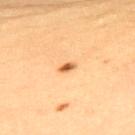Impression:
Captured during whole-body skin photography for melanoma surveillance; the lesion was not biopsied.
Background:
The subject is a female roughly 35 years of age. From the upper back. Approximately 2 mm at its widest. Automated tile analysis of the lesion measured an area of roughly 2 mm² and an outline eccentricity of about 0.85 (0 = round, 1 = elongated). It also reported an average lesion color of about L≈63 a*≈25 b*≈45 (CIELAB), roughly 16 lightness units darker than nearby skin, and a normalized lesion–skin contrast near 9.5. And it measured a within-lesion color-variation index near 2/10 and a peripheral color-asymmetry measure near 1. The analysis additionally found an automated nevus-likeness rating near 100 out of 100 and a lesion-detection confidence of about 100/100. Captured under cross-polarized illumination. A 15 mm close-up extracted from a 3D total-body photography capture.